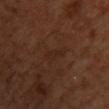  biopsy_status: not biopsied; imaged during a skin examination
  lighting: cross-polarized
  site: chest
  patient:
    sex: female
    age_approx: 55
  automated_metrics:
    eccentricity: 0.95
    shape_asymmetry: 0.3
    cielab_L: 25
    cielab_a: 20
    cielab_b: 25
    vs_skin_darker_L: 4.0
    vs_skin_contrast_norm: 4.5
    border_irregularity_0_10: 4.0
    color_variation_0_10: 0.5
    peripheral_color_asymmetry: 0.0
    nevus_likeness_0_100: 0
  lesion_size:
    long_diameter_mm_approx: 3.5
  image:
    source: total-body photography crop
    field_of_view_mm: 15